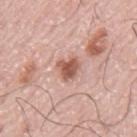Q: Is there a histopathology result?
A: total-body-photography surveillance lesion; no biopsy
Q: What is the lesion's diameter?
A: ≈2.5 mm
Q: Illumination type?
A: white-light
Q: Patient demographics?
A: male, aged 73 to 77
Q: What is the imaging modality?
A: ~15 mm crop, total-body skin-cancer survey
Q: Lesion location?
A: the mid back
Q: What did automated image analysis measure?
A: border irregularity of about 3.5 on a 0–10 scale, internal color variation of about 4 on a 0–10 scale, and radial color variation of about 1; a detector confidence of about 100 out of 100 that the crop contains a lesion